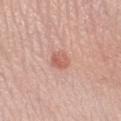follow-up=no biopsy performed (imaged during a skin exam); patient=female, aged 43–47; imaging modality=total-body-photography crop, ~15 mm field of view; anatomic site=the right lower leg; lighting=white-light; lesion diameter=~2.5 mm (longest diameter).